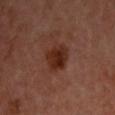Imaged during a routine full-body skin examination; the lesion was not biopsied and no histopathology is available. The lesion's longest dimension is about 3.5 mm. The subject is a male approximately 50 years of age. The lesion is on the chest. Captured under cross-polarized illumination. A roughly 15 mm field-of-view crop from a total-body skin photograph. The lesion-visualizer software estimated a lesion area of about 8 mm², an eccentricity of roughly 0.45, and a shape-asymmetry score of about 0.2 (0 = symmetric). The analysis additionally found a lesion color around L≈27 a*≈23 b*≈26 in CIELAB and a lesion–skin lightness drop of about 9.